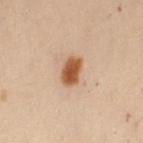{
  "patient": {
    "sex": "female",
    "age_approx": 50
  },
  "image": {
    "source": "total-body photography crop",
    "field_of_view_mm": 15
  },
  "lighting": "cross-polarized",
  "site": "abdomen"
}